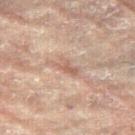<record>
<biopsy_status>not biopsied; imaged during a skin examination</biopsy_status>
<site>leg</site>
<patient>
  <sex>female</sex>
  <age_approx>80</age_approx>
</patient>
<lesion_size>
  <long_diameter_mm_approx>3.5</long_diameter_mm_approx>
</lesion_size>
<lighting>cross-polarized</lighting>
<image>
  <source>total-body photography crop</source>
  <field_of_view_mm>15</field_of_view_mm>
</image>
</record>